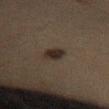workup = no biopsy performed (imaged during a skin exam)
location = the front of the torso
image = ~15 mm crop, total-body skin-cancer survey
patient = female, roughly 60 years of age